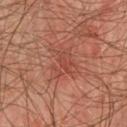Notes:
* biopsy status · total-body-photography surveillance lesion; no biopsy
* image source · total-body-photography crop, ~15 mm field of view
* illumination · cross-polarized illumination
* lesion diameter · ≈3.5 mm
* patient · male, aged approximately 65
* location · the chest